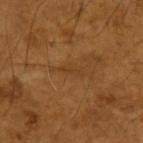Assessment:
The lesion was photographed on a routine skin check and not biopsied; there is no pathology result.
Background:
The total-body-photography lesion software estimated a lesion area of about 2 mm² and an eccentricity of roughly 0.95. It also reported border irregularity of about 4.5 on a 0–10 scale, internal color variation of about 0 on a 0–10 scale, and peripheral color asymmetry of about 0. The analysis additionally found lesion-presence confidence of about 50/100. A close-up tile cropped from a whole-body skin photograph, about 15 mm across. From the left upper arm. A male subject aged around 65. The tile uses cross-polarized illumination. The lesion's longest dimension is about 2.5 mm.